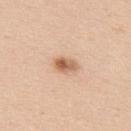| key | value |
|---|---|
| notes | imaged on a skin check; not biopsied |
| subject | male, roughly 40 years of age |
| image-analysis metrics | border irregularity of about 2 on a 0–10 scale, a color-variation rating of about 6/10, and a peripheral color-asymmetry measure near 2; a nevus-likeness score of about 95/100 and lesion-presence confidence of about 100/100 |
| illumination | white-light illumination |
| site | the upper back |
| imaging modality | ~15 mm crop, total-body skin-cancer survey |